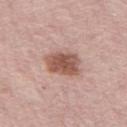<lesion>
<biopsy_status>not biopsied; imaged during a skin examination</biopsy_status>
<lighting>white-light</lighting>
<image>
  <source>total-body photography crop</source>
  <field_of_view_mm>15</field_of_view_mm>
</image>
<site>leg</site>
<patient>
  <sex>female</sex>
  <age_approx>65</age_approx>
</patient>
</lesion>